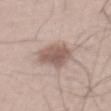No biopsy was performed on this lesion — it was imaged during a full skin examination and was not determined to be concerning.
This image is a 15 mm lesion crop taken from a total-body photograph.
The lesion-visualizer software estimated a lesion area of about 12 mm², a shape eccentricity near 0.75, and a shape-asymmetry score of about 0.2 (0 = symmetric). The analysis additionally found a nevus-likeness score of about 80/100 and lesion-presence confidence of about 100/100.
This is a white-light tile.
The subject is a male roughly 55 years of age.
About 5 mm across.